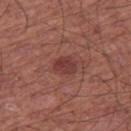workup: no biopsy performed (imaged during a skin exam)
image: ~15 mm crop, total-body skin-cancer survey
size: about 2.5 mm
site: the right thigh
illumination: white-light
subject: male, in their mid-60s